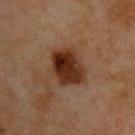– follow-up · imaged on a skin check; not biopsied
– automated lesion analysis · a lesion area of about 14 mm², an eccentricity of roughly 0.35, and two-axis asymmetry of about 0.15
– site · the chest
– acquisition · total-body-photography crop, ~15 mm field of view
– subject · male, aged 63 to 67
– diameter · ≈4 mm
– lighting · cross-polarized